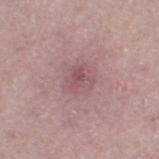Recorded during total-body skin imaging; not selected for excision or biopsy. The lesion is on the left thigh. Measured at roughly 3 mm in maximum diameter. The subject is a male aged 53 to 57. A 15 mm crop from a total-body photograph taken for skin-cancer surveillance. This is a white-light tile.